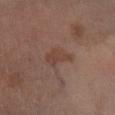- workup · imaged on a skin check; not biopsied
- TBP lesion metrics · an average lesion color of about L≈39 a*≈18 b*≈24 (CIELAB), about 6 CIELAB-L* units darker than the surrounding skin, and a normalized lesion–skin contrast near 5.5; a border-irregularity rating of about 4/10 and a within-lesion color-variation index near 1.5/10; a nevus-likeness score of about 0/100 and lesion-presence confidence of about 100/100
- diameter · about 3.5 mm
- image source · ~15 mm tile from a whole-body skin photo
- location · the left leg
- subject · male, aged approximately 65